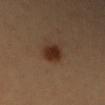Impression:
The lesion was photographed on a routine skin check and not biopsied; there is no pathology result.
Background:
Captured under cross-polarized illumination. A female subject aged 33–37. Located on the right upper arm. The lesion's longest dimension is about 3.5 mm. This image is a 15 mm lesion crop taken from a total-body photograph.